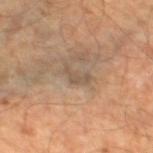Imaged during a routine full-body skin examination; the lesion was not biopsied and no histopathology is available.
Located on the left thigh.
The subject is a male aged around 55.
A lesion tile, about 15 mm wide, cut from a 3D total-body photograph.
The recorded lesion diameter is about 3 mm.